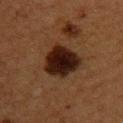Clinical impression:
The lesion was photographed on a routine skin check and not biopsied; there is no pathology result.
Acquisition and patient details:
The patient is a female in their 40s. A 15 mm crop from a total-body photograph taken for skin-cancer surveillance. The lesion is on the upper back.